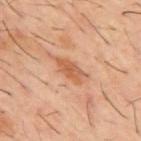follow-up: no biopsy performed (imaged during a skin exam) | acquisition: 15 mm crop, total-body photography | site: the mid back | lighting: cross-polarized | lesion size: about 4 mm | image-analysis metrics: a mean CIELAB color near L≈54 a*≈23 b*≈35, a lesion–skin lightness drop of about 9, and a lesion-to-skin contrast of about 7 (normalized; higher = more distinct); a border-irregularity index near 3.5/10 and internal color variation of about 2.5 on a 0–10 scale | patient: male, aged 58–62.